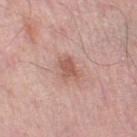lighting — white-light illumination
automated metrics — an eccentricity of roughly 0.75 and a shape-asymmetry score of about 0.3 (0 = symmetric); a mean CIELAB color near L≈56 a*≈23 b*≈27, a lesion–skin lightness drop of about 10, and a lesion-to-skin contrast of about 7 (normalized; higher = more distinct); border irregularity of about 3.5 on a 0–10 scale, a within-lesion color-variation index near 1.5/10, and a peripheral color-asymmetry measure near 0.5; a nevus-likeness score of about 35/100 and a detector confidence of about 100 out of 100 that the crop contains a lesion
patient — male, in their mid- to late 50s
body site — the right lower leg
image source — ~15 mm tile from a whole-body skin photo
size — about 3 mm